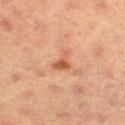Q: Was this lesion biopsied?
A: total-body-photography surveillance lesion; no biopsy
Q: Where on the body is the lesion?
A: the right thigh
Q: Who is the patient?
A: female, aged approximately 55
Q: What is the imaging modality?
A: ~15 mm tile from a whole-body skin photo
Q: How large is the lesion?
A: ≈3 mm
Q: Illumination type?
A: cross-polarized illumination